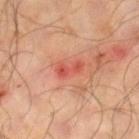Impression: Part of a total-body skin-imaging series; this lesion was reviewed on a skin check and was not flagged for biopsy. Acquisition and patient details: A 15 mm close-up tile from a total-body photography series done for melanoma screening. Imaged with cross-polarized lighting. A male patient, aged approximately 65. The recorded lesion diameter is about 3 mm. The lesion is on the right lower leg.